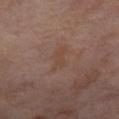biopsy_status: not biopsied; imaged during a skin examination
site: leg
lesion_size:
  long_diameter_mm_approx: 3.5
lighting: cross-polarized
image:
  source: total-body photography crop
  field_of_view_mm: 15
patient:
  sex: female
  age_approx: 55
automated_metrics:
  border_irregularity_0_10: 6.0
  color_variation_0_10: 0.5
  peripheral_color_asymmetry: 0.0